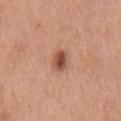Case summary:
• biopsy status — no biopsy performed (imaged during a skin exam)
• image-analysis metrics — internal color variation of about 6 on a 0–10 scale
• size — about 3 mm
• tile lighting — white-light illumination
• patient — male, approximately 70 years of age
• location — the chest
• image — ~15 mm crop, total-body skin-cancer survey